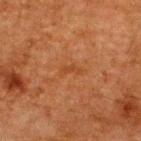<tbp_lesion>
<biopsy_status>not biopsied; imaged during a skin examination</biopsy_status>
<image>
  <source>total-body photography crop</source>
  <field_of_view_mm>15</field_of_view_mm>
</image>
<patient>
  <sex>male</sex>
  <age_approx>60</age_approx>
</patient>
<lighting>cross-polarized</lighting>
<lesion_size>
  <long_diameter_mm_approx>2.5</long_diameter_mm_approx>
</lesion_size>
<automated_metrics>
  <vs_skin_darker_L>4.0</vs_skin_darker_L>
  <border_irregularity_0_10>4.0</border_irregularity_0_10>
  <color_variation_0_10>0.0</color_variation_0_10>
  <peripheral_color_asymmetry>0.0</peripheral_color_asymmetry>
  <nevus_likeness_0_100>0</nevus_likeness_0_100>
  <lesion_detection_confidence_0_100>100</lesion_detection_confidence_0_100>
</automated_metrics>
<site>upper back</site>
</tbp_lesion>